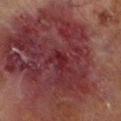Clinical impression: This lesion was catalogued during total-body skin photography and was not selected for biopsy. Image and clinical context: Captured under cross-polarized illumination. About 2 mm across. The lesion is located on the left lower leg. A male patient, aged around 70. A 15 mm crop from a total-body photograph taken for skin-cancer surveillance.